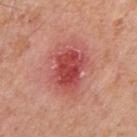The lesion was tiled from a total-body skin photograph and was not biopsied.
The recorded lesion diameter is about 6 mm.
Cropped from a whole-body photographic skin survey; the tile spans about 15 mm.
A male patient aged around 55.
This is a white-light tile.
The lesion is on the arm.